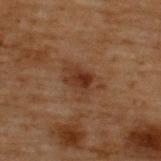Impression:
Recorded during total-body skin imaging; not selected for excision or biopsy.
Background:
A region of skin cropped from a whole-body photographic capture, roughly 15 mm wide. On the upper back. A male patient aged 68–72. Automated tile analysis of the lesion measured a border-irregularity index near 4/10, a within-lesion color-variation index near 4/10, and radial color variation of about 1.